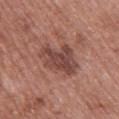The lesion's longest dimension is about 5 mm.
This is a white-light tile.
The lesion is located on the upper back.
A lesion tile, about 15 mm wide, cut from a 3D total-body photograph.
The patient is a female aged 58 to 62.